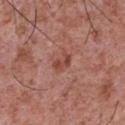Captured during whole-body skin photography for melanoma surveillance; the lesion was not biopsied. From the chest. The recorded lesion diameter is about 2.5 mm. The tile uses white-light illumination. A male subject, roughly 45 years of age. A region of skin cropped from a whole-body photographic capture, roughly 15 mm wide. The total-body-photography lesion software estimated an average lesion color of about L≈46 a*≈26 b*≈27 (CIELAB) and roughly 9 lightness units darker than nearby skin. The analysis additionally found a color-variation rating of about 2.5/10 and a peripheral color-asymmetry measure near 0.5. And it measured a detector confidence of about 100 out of 100 that the crop contains a lesion.